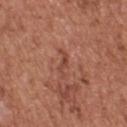The lesion was photographed on a routine skin check and not biopsied; there is no pathology result.
The recorded lesion diameter is about 3.5 mm.
A male subject, in their mid- to late 40s.
Automated image analysis of the tile measured a lesion area of about 3.5 mm² and two-axis asymmetry of about 0.7. It also reported an average lesion color of about L≈46 a*≈25 b*≈30 (CIELAB) and a lesion–skin lightness drop of about 7. It also reported an automated nevus-likeness rating near 0 out of 100 and a detector confidence of about 85 out of 100 that the crop contains a lesion.
Captured under white-light illumination.
On the chest.
A lesion tile, about 15 mm wide, cut from a 3D total-body photograph.